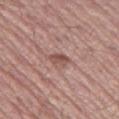Notes:
• biopsy status · catalogued during a skin exam; not biopsied
• image source · total-body-photography crop, ~15 mm field of view
• body site · the left thigh
• TBP lesion metrics · a footprint of about 3.5 mm² and two-axis asymmetry of about 0.3; a lesion color around L≈51 a*≈21 b*≈23 in CIELAB; an automated nevus-likeness rating near 0 out of 100 and a lesion-detection confidence of about 95/100
• patient · male, roughly 55 years of age